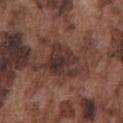notes=imaged on a skin check; not biopsied
patient=male, approximately 75 years of age
imaging modality=15 mm crop, total-body photography
site=the chest